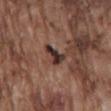Q: How was the tile lit?
A: white-light
Q: What is the anatomic site?
A: the mid back
Q: What kind of image is this?
A: total-body-photography crop, ~15 mm field of view
Q: What are the patient's age and sex?
A: male, aged 73 to 77
Q: What did automated image analysis measure?
A: a shape eccentricity near 0.8; roughly 15 lightness units darker than nearby skin and a normalized border contrast of about 13.5